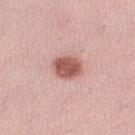| feature | finding |
|---|---|
| biopsy status | catalogued during a skin exam; not biopsied |
| body site | the left lower leg |
| image | ~15 mm crop, total-body skin-cancer survey |
| patient | female, aged approximately 35 |